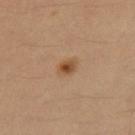follow-up: no biopsy performed (imaged during a skin exam) | size: ≈2 mm | location: the right thigh | patient: male, in their 30s | TBP lesion metrics: an average lesion color of about L≈50 a*≈20 b*≈36 (CIELAB), roughly 11 lightness units darker than nearby skin, and a normalized lesion–skin contrast near 8.5; a nevus-likeness score of about 95/100 and a lesion-detection confidence of about 100/100 | tile lighting: cross-polarized illumination | image: ~15 mm tile from a whole-body skin photo.